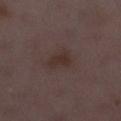notes: imaged on a skin check; not biopsied
subject: female, aged 28–32
image: ~15 mm tile from a whole-body skin photo
body site: the leg